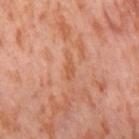Part of a total-body skin-imaging series; this lesion was reviewed on a skin check and was not flagged for biopsy. A female subject, aged 53–57. Cropped from a total-body skin-imaging series; the visible field is about 15 mm. On the right thigh.